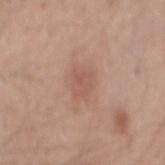Q: Was this lesion biopsied?
A: no biopsy performed (imaged during a skin exam)
Q: What is the imaging modality?
A: ~15 mm crop, total-body skin-cancer survey
Q: Where on the body is the lesion?
A: the mid back
Q: Lesion size?
A: ~4 mm (longest diameter)
Q: What did automated image analysis measure?
A: an eccentricity of roughly 0.75; a lesion–skin lightness drop of about 7 and a normalized lesion–skin contrast near 5
Q: Patient demographics?
A: male, in their mid-40s
Q: What lighting was used for the tile?
A: white-light